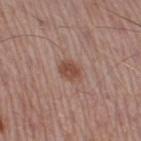notes=no biopsy performed (imaged during a skin exam); site=the right thigh; patient=male, roughly 55 years of age; image source=~15 mm tile from a whole-body skin photo; lighting=white-light illumination.